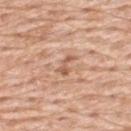Imaged during a routine full-body skin examination; the lesion was not biopsied and no histopathology is available. A close-up tile cropped from a whole-body skin photograph, about 15 mm across. Captured under white-light illumination. Located on the back. Automated image analysis of the tile measured a lesion area of about 3 mm², an eccentricity of roughly 0.85, and two-axis asymmetry of about 0.55. The software also gave roughly 10 lightness units darker than nearby skin. And it measured a nevus-likeness score of about 0/100 and lesion-presence confidence of about 100/100. The patient is a male aged 58–62.